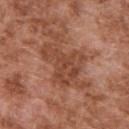This lesion was catalogued during total-body skin photography and was not selected for biopsy.
This image is a 15 mm lesion crop taken from a total-body photograph.
The lesion is located on the upper back.
A male subject aged 73–77.